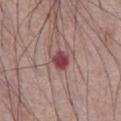A male patient in their mid-60s. The lesion's longest dimension is about 2.5 mm. Cropped from a whole-body photographic skin survey; the tile spans about 15 mm. This is a white-light tile. From the abdomen. The total-body-photography lesion software estimated a shape-asymmetry score of about 0.2 (0 = symmetric). And it measured an average lesion color of about L≈44 a*≈28 b*≈19 (CIELAB). And it measured a border-irregularity rating of about 1.5/10, internal color variation of about 3.5 on a 0–10 scale, and a peripheral color-asymmetry measure near 1.5. It also reported a classifier nevus-likeness of about 0/100 and a lesion-detection confidence of about 100/100.